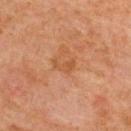The lesion was photographed on a routine skin check and not biopsied; there is no pathology result. From the upper back. Imaged with cross-polarized lighting. A female patient, in their 70s. The lesion's longest dimension is about 2.5 mm. A 15 mm close-up extracted from a 3D total-body photography capture.